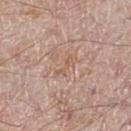Case summary:
• follow-up · no biopsy performed (imaged during a skin exam)
• tile lighting · white-light illumination
• body site · the leg
• lesion size · ~3 mm (longest diameter)
• subject · male, aged 63 to 67
• imaging modality · 15 mm crop, total-body photography